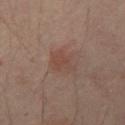biopsy_status: not biopsied; imaged during a skin examination
image:
  source: total-body photography crop
  field_of_view_mm: 15
lesion_size:
  long_diameter_mm_approx: 3.5
lighting: cross-polarized
site: abdomen
patient:
  sex: male
  age_approx: 60